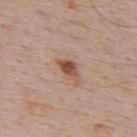Q: Is there a histopathology result?
A: imaged on a skin check; not biopsied
Q: Patient demographics?
A: male, approximately 70 years of age
Q: Lesion location?
A: the upper back
Q: How was this image acquired?
A: 15 mm crop, total-body photography
Q: Illumination type?
A: white-light illumination
Q: What did automated image analysis measure?
A: a border-irregularity index near 3.5/10, internal color variation of about 5 on a 0–10 scale, and radial color variation of about 2; a nevus-likeness score of about 90/100